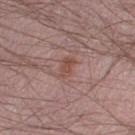biopsy status: catalogued during a skin exam; not biopsied
location: the right thigh
image source: ~15 mm crop, total-body skin-cancer survey
patient: male, approximately 25 years of age